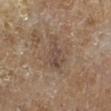Findings:
- follow-up · imaged on a skin check; not biopsied
- lesion diameter · ≈4.5 mm
- body site · the left lower leg
- acquisition · 15 mm crop, total-body photography
- image-analysis metrics · an area of roughly 8 mm² and an eccentricity of roughly 0.75; a lesion color around L≈44 a*≈14 b*≈23 in CIELAB, about 7 CIELAB-L* units darker than the surrounding skin, and a normalized border contrast of about 6; a border-irregularity index near 5/10, a within-lesion color-variation index near 3.5/10, and a peripheral color-asymmetry measure near 1.5
- patient · male, about 85 years old or older